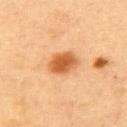notes: no biopsy performed (imaged during a skin exam); patient: female, about 60 years old; anatomic site: the upper back; lesion diameter: ≈4 mm; lighting: cross-polarized illumination; image source: total-body-photography crop, ~15 mm field of view.